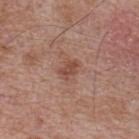biopsy status: catalogued during a skin exam; not biopsied | body site: the upper back | patient: male, aged approximately 65 | imaging modality: 15 mm crop, total-body photography | illumination: white-light illumination | automated lesion analysis: a shape eccentricity near 0.75 and two-axis asymmetry of about 0.35; an average lesion color of about L≈48 a*≈22 b*≈27 (CIELAB), about 9 CIELAB-L* units darker than the surrounding skin, and a normalized lesion–skin contrast near 7; internal color variation of about 1 on a 0–10 scale and a peripheral color-asymmetry measure near 0.5; an automated nevus-likeness rating near 0 out of 100 and a lesion-detection confidence of about 100/100 | lesion diameter: ≈2.5 mm.